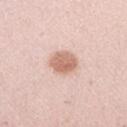follow-up — imaged on a skin check; not biopsied
subject — female, approximately 30 years of age
image source — ~15 mm crop, total-body skin-cancer survey
diameter — about 3.5 mm
lighting — white-light
body site — the right forearm